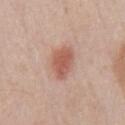Case summary:
– workup — total-body-photography surveillance lesion; no biopsy
– size — ≈4.5 mm
– patient — male, aged around 60
– image — 15 mm crop, total-body photography
– illumination — white-light
– image-analysis metrics — a mean CIELAB color near L≈57 a*≈24 b*≈28, roughly 11 lightness units darker than nearby skin, and a lesion-to-skin contrast of about 8 (normalized; higher = more distinct); a border-irregularity rating of about 2/10
– body site — the chest